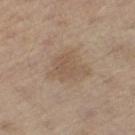follow-up: no biopsy performed (imaged during a skin exam)
lesion size: ~5 mm (longest diameter)
anatomic site: the leg
image-analysis metrics: a border-irregularity rating of about 4/10, a color-variation rating of about 2/10, and radial color variation of about 0.5
subject: male, in their 70s
image: 15 mm crop, total-body photography